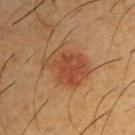Captured during whole-body skin photography for melanoma surveillance; the lesion was not biopsied.
A close-up tile cropped from a whole-body skin photograph, about 15 mm across.
A male subject roughly 35 years of age.
On the right upper arm.